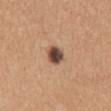biopsy status: total-body-photography surveillance lesion; no biopsy | subject: female, about 40 years old | image: ~15 mm tile from a whole-body skin photo | lighting: white-light illumination | location: the mid back | lesion size: ~2.5 mm (longest diameter).